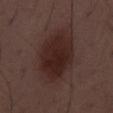Image and clinical context: The tile uses white-light illumination. A close-up tile cropped from a whole-body skin photograph, about 15 mm across. A male subject, aged approximately 50.